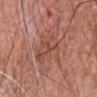Q: Is there a histopathology result?
A: total-body-photography surveillance lesion; no biopsy
Q: Where on the body is the lesion?
A: the head or neck
Q: Patient demographics?
A: male, roughly 65 years of age
Q: What is the imaging modality?
A: ~15 mm crop, total-body skin-cancer survey
Q: What did automated image analysis measure?
A: an average lesion color of about L≈51 a*≈24 b*≈27 (CIELAB) and a normalized lesion–skin contrast near 5; border irregularity of about 5.5 on a 0–10 scale, a within-lesion color-variation index near 5/10, and peripheral color asymmetry of about 2; a nevus-likeness score of about 0/100 and lesion-presence confidence of about 90/100
Q: Illumination type?
A: white-light illumination
Q: What is the lesion's diameter?
A: about 7.5 mm